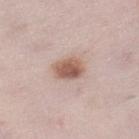Assessment:
The lesion was photographed on a routine skin check and not biopsied; there is no pathology result.
Background:
A region of skin cropped from a whole-body photographic capture, roughly 15 mm wide. A female subject, aged 28 to 32. On the left lower leg.